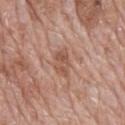  biopsy_status: not biopsied; imaged during a skin examination
  automated_metrics:
    eccentricity: 0.8
    shape_asymmetry: 0.3
    nevus_likeness_0_100: 0
    lesion_detection_confidence_0_100: 100
  image:
    source: total-body photography crop
    field_of_view_mm: 15
  lighting: white-light
  site: mid back
  lesion_size:
    long_diameter_mm_approx: 4.0
  patient:
    sex: male
    age_approx: 70